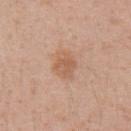Clinical impression: Part of a total-body skin-imaging series; this lesion was reviewed on a skin check and was not flagged for biopsy.